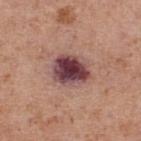<record>
  <biopsy_status>not biopsied; imaged during a skin examination</biopsy_status>
  <lesion_size>
    <long_diameter_mm_approx>4.5</long_diameter_mm_approx>
  </lesion_size>
  <patient>
    <sex>male</sex>
    <age_approx>70</age_approx>
  </patient>
  <lighting>white-light</lighting>
  <site>upper back</site>
  <image>
    <source>total-body photography crop</source>
    <field_of_view_mm>15</field_of_view_mm>
  </image>
  <automated_metrics>
    <area_mm2_approx>13.0</area_mm2_approx>
    <eccentricity>0.6</eccentricity>
    <shape_asymmetry>0.15</shape_asymmetry>
  </automated_metrics>
</record>